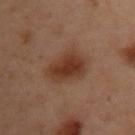| field | value |
|---|---|
| biopsy status | no biopsy performed (imaged during a skin exam) |
| location | the left arm |
| imaging modality | ~15 mm crop, total-body skin-cancer survey |
| subject | female, roughly 55 years of age |
| tile lighting | cross-polarized |
| lesion diameter | ≈5 mm |